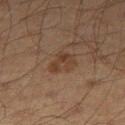biopsy status = total-body-photography surveillance lesion; no biopsy | illumination = cross-polarized | imaging modality = ~15 mm tile from a whole-body skin photo | lesion diameter = ~3.5 mm (longest diameter) | anatomic site = the right thigh | patient = male, aged approximately 60.